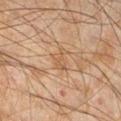This lesion was catalogued during total-body skin photography and was not selected for biopsy. Measured at roughly 2.5 mm in maximum diameter. Imaged with cross-polarized lighting. The patient is a male about 45 years old. Automated image analysis of the tile measured a lesion–skin lightness drop of about 5 and a normalized border contrast of about 5. And it measured a border-irregularity index near 4/10, a color-variation rating of about 1.5/10, and peripheral color asymmetry of about 0.5. It also reported a nevus-likeness score of about 0/100 and a detector confidence of about 55 out of 100 that the crop contains a lesion. Located on the right lower leg. A lesion tile, about 15 mm wide, cut from a 3D total-body photograph.